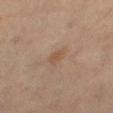Q: Was this lesion biopsied?
A: no biopsy performed (imaged during a skin exam)
Q: What are the patient's age and sex?
A: male, aged approximately 60
Q: Where on the body is the lesion?
A: the right thigh
Q: How was this image acquired?
A: total-body-photography crop, ~15 mm field of view
Q: How was the tile lit?
A: cross-polarized illumination
Q: How large is the lesion?
A: ~3 mm (longest diameter)